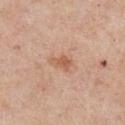{
  "biopsy_status": "not biopsied; imaged during a skin examination",
  "patient": {
    "sex": "male",
    "age_approx": 55
  },
  "lighting": "white-light",
  "site": "chest",
  "automated_metrics": {
    "area_mm2_approx": 4.5,
    "eccentricity": 0.85,
    "shape_asymmetry": 0.3,
    "cielab_L": 59,
    "cielab_a": 23,
    "cielab_b": 32,
    "vs_skin_darker_L": 9.0,
    "vs_skin_contrast_norm": 6.5,
    "border_irregularity_0_10": 3.0,
    "color_variation_0_10": 2.5,
    "peripheral_color_asymmetry": 1.0
  },
  "lesion_size": {
    "long_diameter_mm_approx": 3.0
  },
  "image": {
    "source": "total-body photography crop",
    "field_of_view_mm": 15
  }
}